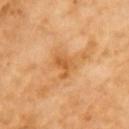Q: Was a biopsy performed?
A: catalogued during a skin exam; not biopsied
Q: Automated lesion metrics?
A: a lesion color around L≈60 a*≈26 b*≈47 in CIELAB; internal color variation of about 2 on a 0–10 scale
Q: How was this image acquired?
A: ~15 mm tile from a whole-body skin photo
Q: Patient demographics?
A: female, aged approximately 70
Q: What is the anatomic site?
A: the right upper arm